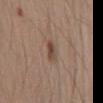No biopsy was performed on this lesion — it was imaged during a full skin examination and was not determined to be concerning. A male patient, aged 38 to 42. A roughly 15 mm field-of-view crop from a total-body skin photograph. The lesion-visualizer software estimated a lesion area of about 3.5 mm², an eccentricity of roughly 0.9, and a symmetry-axis asymmetry near 0.3. It also reported an average lesion color of about L≈46 a*≈16 b*≈25 (CIELAB) and roughly 10 lightness units darker than nearby skin. It also reported a border-irregularity index near 3/10 and radial color variation of about 0.5. About 3 mm across. Captured under white-light illumination. Located on the mid back.